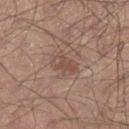Assessment: Captured during whole-body skin photography for melanoma surveillance; the lesion was not biopsied.